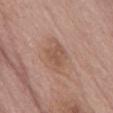notes = imaged on a skin check; not biopsied | illumination = white-light illumination | size = about 3 mm | subject = male, in their 70s | site = the abdomen | imaging modality = ~15 mm crop, total-body skin-cancer survey.